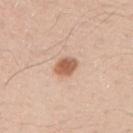Measured at roughly 2.5 mm in maximum diameter. A male subject roughly 30 years of age. The total-body-photography lesion software estimated an average lesion color of about L≈60 a*≈22 b*≈32 (CIELAB), roughly 14 lightness units darker than nearby skin, and a lesion-to-skin contrast of about 9 (normalized; higher = more distinct). The software also gave a nevus-likeness score of about 100/100 and a detector confidence of about 100 out of 100 that the crop contains a lesion. This is a white-light tile. A close-up tile cropped from a whole-body skin photograph, about 15 mm across. The lesion is on the right upper arm.